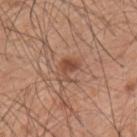Impression:
The lesion was tiled from a total-body skin photograph and was not biopsied.
Acquisition and patient details:
Cropped from a whole-body photographic skin survey; the tile spans about 15 mm. Captured under white-light illumination. Measured at roughly 3 mm in maximum diameter. A male subject roughly 60 years of age. The lesion is on the left upper arm.